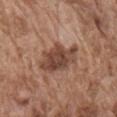Clinical impression: Captured during whole-body skin photography for melanoma surveillance; the lesion was not biopsied. Acquisition and patient details: Located on the chest. Automated tile analysis of the lesion measured an area of roughly 13 mm², an outline eccentricity of about 0.7 (0 = round, 1 = elongated), and two-axis asymmetry of about 0.25. It also reported a mean CIELAB color near L≈45 a*≈20 b*≈27 and a normalized lesion–skin contrast near 9. The software also gave lesion-presence confidence of about 100/100. A lesion tile, about 15 mm wide, cut from a 3D total-body photograph. The patient is a male approximately 75 years of age. This is a white-light tile. Approximately 5 mm at its widest.